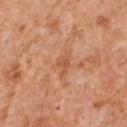notes: no biopsy performed (imaged during a skin exam) | lighting: white-light illumination | subject: male, in their mid-50s | image: 15 mm crop, total-body photography | diameter: ~3.5 mm (longest diameter) | location: the chest | automated lesion analysis: an average lesion color of about L≈56 a*≈26 b*≈37 (CIELAB) and about 7 CIELAB-L* units darker than the surrounding skin; border irregularity of about 4.5 on a 0–10 scale and internal color variation of about 1.5 on a 0–10 scale; lesion-presence confidence of about 100/100.